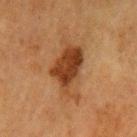Assessment:
This lesion was catalogued during total-body skin photography and was not selected for biopsy.
Acquisition and patient details:
A female patient, aged 53 to 57. The lesion is on the left upper arm. Automated tile analysis of the lesion measured an outline eccentricity of about 0.85 (0 = round, 1 = elongated) and two-axis asymmetry of about 0.35. It also reported a lesion color around L≈35 a*≈20 b*≈31 in CIELAB, about 11 CIELAB-L* units darker than the surrounding skin, and a lesion-to-skin contrast of about 9.5 (normalized; higher = more distinct). It also reported a border-irregularity rating of about 4.5/10, a within-lesion color-variation index near 6/10, and radial color variation of about 2. It also reported a classifier nevus-likeness of about 95/100 and a detector confidence of about 100 out of 100 that the crop contains a lesion. A 15 mm crop from a total-body photograph taken for skin-cancer surveillance. Measured at roughly 7 mm in maximum diameter. Imaged with cross-polarized lighting.